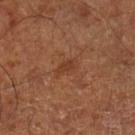Clinical impression:
This lesion was catalogued during total-body skin photography and was not selected for biopsy.
Clinical summary:
The patient is approximately 65 years of age. An algorithmic analysis of the crop reported an area of roughly 3.5 mm² and a shape eccentricity near 0.85. And it measured border irregularity of about 2 on a 0–10 scale, a color-variation rating of about 1/10, and a peripheral color-asymmetry measure near 0.5. And it measured an automated nevus-likeness rating near 0 out of 100 and lesion-presence confidence of about 100/100. The recorded lesion diameter is about 3 mm. On the leg. Cropped from a total-body skin-imaging series; the visible field is about 15 mm.